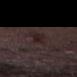Recorded during total-body skin imaging; not selected for excision or biopsy.
A region of skin cropped from a whole-body photographic capture, roughly 15 mm wide.
The subject is a male about 50 years old.
The recorded lesion diameter is about 3 mm.
The lesion is on the right thigh.